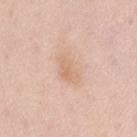The lesion was tiled from a total-body skin photograph and was not biopsied. Located on the lower back. The lesion's longest dimension is about 4.5 mm. The tile uses white-light illumination. A female patient, aged around 65. A 15 mm close-up tile from a total-body photography series done for melanoma screening. An algorithmic analysis of the crop reported a lesion area of about 9 mm². It also reported a border-irregularity index near 2.5/10, internal color variation of about 3 on a 0–10 scale, and a peripheral color-asymmetry measure near 1.